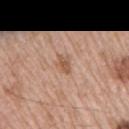The subject is a male about 65 years old. Cropped from a total-body skin-imaging series; the visible field is about 15 mm. Imaged with white-light lighting. The lesion's longest dimension is about 2.5 mm. The lesion is located on the right upper arm.